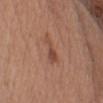Context:
The lesion is on the left upper arm. About 3.5 mm across. Cropped from a whole-body photographic skin survey; the tile spans about 15 mm. Captured under white-light illumination. A male patient in their mid-50s. An algorithmic analysis of the crop reported an average lesion color of about L≈47 a*≈22 b*≈29 (CIELAB) and a lesion-to-skin contrast of about 6.5 (normalized; higher = more distinct). The analysis additionally found a lesion-detection confidence of about 100/100.